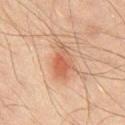The lesion was photographed on a routine skin check and not biopsied; there is no pathology result.
On the chest.
A male subject aged 43–47.
An algorithmic analysis of the crop reported a footprint of about 8.5 mm², a shape eccentricity near 0.8, and a symmetry-axis asymmetry near 0.3. And it measured a mean CIELAB color near L≈49 a*≈21 b*≈29 and a lesion-to-skin contrast of about 6.5 (normalized; higher = more distinct). And it measured a border-irregularity rating of about 3.5/10, a color-variation rating of about 4.5/10, and radial color variation of about 1.5. The software also gave an automated nevus-likeness rating near 85 out of 100 and lesion-presence confidence of about 100/100.
Captured under cross-polarized illumination.
A lesion tile, about 15 mm wide, cut from a 3D total-body photograph.
Longest diameter approximately 4.5 mm.